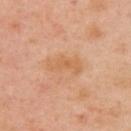* workup · total-body-photography surveillance lesion; no biopsy
* image · ~15 mm crop, total-body skin-cancer survey
* subject · female, aged around 40
* body site · the left arm
* lesion diameter · ≈4.5 mm